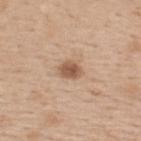biopsy status — no biopsy performed (imaged during a skin exam); anatomic site — the upper back; imaging modality — ~15 mm tile from a whole-body skin photo; patient — male, in their 60s.